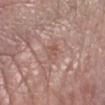Captured during whole-body skin photography for melanoma surveillance; the lesion was not biopsied. The subject is a female approximately 65 years of age. The lesion's longest dimension is about 2.5 mm. A 15 mm close-up tile from a total-body photography series done for melanoma screening. This is a white-light tile. The lesion is located on the arm.